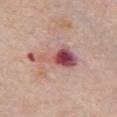Part of a total-body skin-imaging series; this lesion was reviewed on a skin check and was not flagged for biopsy. From the chest. The patient is a female aged 63 to 67. A 15 mm close-up extracted from a 3D total-body photography capture.